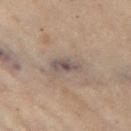| feature | finding |
|---|---|
| workup | catalogued during a skin exam; not biopsied |
| subject | female, approximately 60 years of age |
| tile lighting | cross-polarized illumination |
| imaging modality | 15 mm crop, total-body photography |
| lesion size | ≈3.5 mm |
| location | the left thigh |
| image-analysis metrics | an area of roughly 5 mm² and an eccentricity of roughly 0.85; an average lesion color of about L≈50 a*≈12 b*≈18 (CIELAB) and roughly 9 lightness units darker than nearby skin |